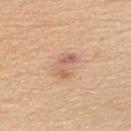follow-up: imaged on a skin check; not biopsied | automated lesion analysis: an automated nevus-likeness rating near 0 out of 100 and a lesion-detection confidence of about 100/100 | subject: male, aged approximately 35 | image source: ~15 mm crop, total-body skin-cancer survey | tile lighting: white-light | body site: the chest | lesion diameter: ≈3.5 mm.